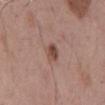Q: Was a biopsy performed?
A: catalogued during a skin exam; not biopsied
Q: What are the patient's age and sex?
A: male, roughly 55 years of age
Q: What kind of image is this?
A: 15 mm crop, total-body photography
Q: Where on the body is the lesion?
A: the mid back
Q: How large is the lesion?
A: ≈3 mm